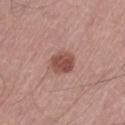Impression: Captured during whole-body skin photography for melanoma surveillance; the lesion was not biopsied. Acquisition and patient details: Located on the left thigh. A 15 mm close-up tile from a total-body photography series done for melanoma screening. A male patient, aged 63 to 67. The lesion's longest dimension is about 3 mm. This is a white-light tile. An algorithmic analysis of the crop reported a lesion color around L≈49 a*≈24 b*≈26 in CIELAB. It also reported border irregularity of about 1 on a 0–10 scale and peripheral color asymmetry of about 1.